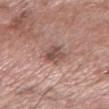Recorded during total-body skin imaging; not selected for excision or biopsy. Located on the right forearm. The patient is a male aged approximately 60. A 15 mm close-up tile from a total-body photography series done for melanoma screening.